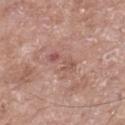Clinical impression: The lesion was photographed on a routine skin check and not biopsied; there is no pathology result. Context: A lesion tile, about 15 mm wide, cut from a 3D total-body photograph. A female subject aged 73 to 77. The lesion is located on the right lower leg.